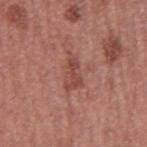Assessment:
The lesion was photographed on a routine skin check and not biopsied; there is no pathology result.
Clinical summary:
Cropped from a whole-body photographic skin survey; the tile spans about 15 mm. On the left upper arm. A male patient approximately 45 years of age.